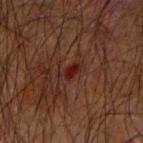<case>
  <biopsy_status>not biopsied; imaged during a skin examination</biopsy_status>
  <image>
    <source>total-body photography crop</source>
    <field_of_view_mm>15</field_of_view_mm>
  </image>
  <lighting>cross-polarized</lighting>
  <site>right upper arm</site>
  <patient>
    <sex>male</sex>
    <age_approx>65</age_approx>
  </patient>
</case>